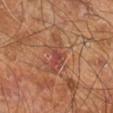A 15 mm crop from a total-body photograph taken for skin-cancer surveillance. A male patient aged 58 to 62. The lesion is on the right leg.